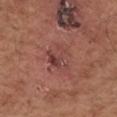| field | value |
|---|---|
| biopsy status | no biopsy performed (imaged during a skin exam) |
| imaging modality | ~15 mm tile from a whole-body skin photo |
| subject | male, roughly 70 years of age |
| illumination | white-light |
| location | the left forearm |